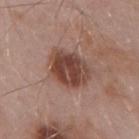follow-up: catalogued during a skin exam; not biopsied | illumination: white-light illumination | imaging modality: total-body-photography crop, ~15 mm field of view | subject: male, aged 53 to 57 | location: the mid back | lesion size: ≈5 mm.